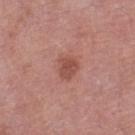Clinical impression: The lesion was tiled from a total-body skin photograph and was not biopsied. Acquisition and patient details: A roughly 15 mm field-of-view crop from a total-body skin photograph. On the left thigh. A female patient approximately 70 years of age.